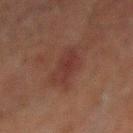Context: Automated tile analysis of the lesion measured an area of roughly 11 mm², an outline eccentricity of about 0.85 (0 = round, 1 = elongated), and a shape-asymmetry score of about 0.3 (0 = symmetric). The software also gave an average lesion color of about L≈27 a*≈18 b*≈19 (CIELAB), roughly 5 lightness units darker than nearby skin, and a lesion-to-skin contrast of about 6 (normalized; higher = more distinct). And it measured border irregularity of about 4 on a 0–10 scale, internal color variation of about 3 on a 0–10 scale, and a peripheral color-asymmetry measure near 1. The analysis additionally found a nevus-likeness score of about 5/100 and a lesion-detection confidence of about 100/100. A male subject about 60 years old. This image is a 15 mm lesion crop taken from a total-body photograph. Measured at roughly 5.5 mm in maximum diameter. Captured under cross-polarized illumination. The lesion is on the mid back.